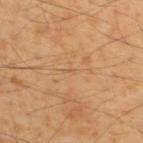| feature | finding |
|---|---|
| follow-up | total-body-photography surveillance lesion; no biopsy |
| site | the upper back |
| lesion diameter | ~1 mm (longest diameter) |
| patient | male, aged 53–57 |
| TBP lesion metrics | a border-irregularity rating of about 2.5/10, a color-variation rating of about 0/10, and radial color variation of about 0 |
| tile lighting | cross-polarized |
| image source | ~15 mm tile from a whole-body skin photo |